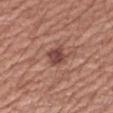No biopsy was performed on this lesion — it was imaged during a full skin examination and was not determined to be concerning. A 15 mm crop from a total-body photograph taken for skin-cancer surveillance. Imaged with white-light lighting. Longest diameter approximately 2.5 mm. The patient is a male aged 58 to 62. Located on the right upper arm.